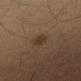Notes:
- follow-up — no biopsy performed (imaged during a skin exam)
- patient — male, about 45 years old
- body site — the right thigh
- image source — ~15 mm crop, total-body skin-cancer survey